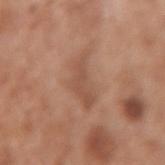lesion diameter: ≈5 mm
lighting: white-light
subject: female, about 50 years old
acquisition: ~15 mm tile from a whole-body skin photo
site: the left forearm